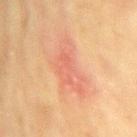Notes:
- notes · imaged on a skin check; not biopsied
- location · the right upper arm
- acquisition · ~15 mm tile from a whole-body skin photo
- subject · female, aged 78–82
- tile lighting · cross-polarized
- lesion diameter · ~7 mm (longest diameter)